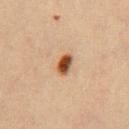The lesion was photographed on a routine skin check and not biopsied; there is no pathology result. The lesion is located on the front of the torso. Approximately 2.5 mm at its widest. A male subject, in their 60s. A 15 mm close-up extracted from a 3D total-body photography capture.